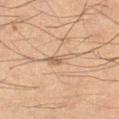The lesion was photographed on a routine skin check and not biopsied; there is no pathology result.
On the right thigh.
A roughly 15 mm field-of-view crop from a total-body skin photograph.
The subject is a male approximately 65 years of age.
The lesion's longest dimension is about 3 mm.